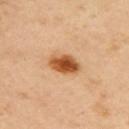{"biopsy_status": "not biopsied; imaged during a skin examination", "image": {"source": "total-body photography crop", "field_of_view_mm": 15}, "patient": {"sex": "female", "age_approx": 45}, "site": "upper back"}